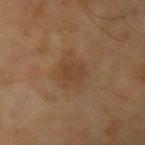Imaged during a routine full-body skin examination; the lesion was not biopsied and no histopathology is available.